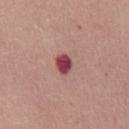Recorded during total-body skin imaging; not selected for excision or biopsy.
On the chest.
A 15 mm crop from a total-body photograph taken for skin-cancer surveillance.
The subject is a female approximately 35 years of age.